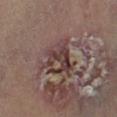workup: imaged on a skin check; not biopsied
location: the left lower leg
imaging modality: total-body-photography crop, ~15 mm field of view
automated lesion analysis: an area of roughly 12 mm², an outline eccentricity of about 0.55 (0 = round, 1 = elongated), and a symmetry-axis asymmetry near 0.35; a nevus-likeness score of about 0/100 and a lesion-detection confidence of about 10/100
patient: male, roughly 70 years of age
lesion diameter: ~4.5 mm (longest diameter)
illumination: cross-polarized